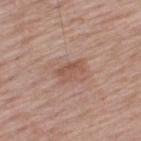follow-up = total-body-photography surveillance lesion; no biopsy | image-analysis metrics = a lesion area of about 4.5 mm², a shape eccentricity near 0.85, and a symmetry-axis asymmetry near 0.35; an automated nevus-likeness rating near 5 out of 100 and a lesion-detection confidence of about 100/100 | lighting = white-light illumination | patient = male, aged 63 to 67 | imaging modality = 15 mm crop, total-body photography | site = the upper back.